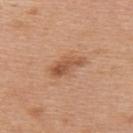Imaged during a routine full-body skin examination; the lesion was not biopsied and no histopathology is available.
A roughly 15 mm field-of-view crop from a total-body skin photograph.
Longest diameter approximately 4.5 mm.
Automated tile analysis of the lesion measured a shape eccentricity near 0.9 and a shape-asymmetry score of about 0.3 (0 = symmetric). And it measured border irregularity of about 3.5 on a 0–10 scale, a color-variation rating of about 4.5/10, and a peripheral color-asymmetry measure near 1.5.
Located on the upper back.
The subject is a female roughly 40 years of age.